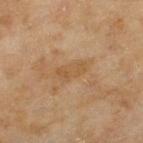{"biopsy_status": "not biopsied; imaged during a skin examination", "patient": {"sex": "female", "age_approx": 60}, "lesion_size": {"long_diameter_mm_approx": 4.0}, "automated_metrics": {"area_mm2_approx": 6.0, "eccentricity": 0.9, "lesion_detection_confidence_0_100": 100}, "image": {"source": "total-body photography crop", "field_of_view_mm": 15}, "lighting": "cross-polarized", "site": "left thigh"}